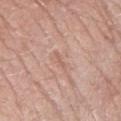Q: Was a biopsy performed?
A: no biopsy performed (imaged during a skin exam)
Q: What lighting was used for the tile?
A: white-light
Q: Where on the body is the lesion?
A: the right forearm
Q: How large is the lesion?
A: ≈2.5 mm
Q: What kind of image is this?
A: total-body-photography crop, ~15 mm field of view
Q: What did automated image analysis measure?
A: an area of roughly 2 mm², an eccentricity of roughly 0.95, and a symmetry-axis asymmetry near 0.45; internal color variation of about 0 on a 0–10 scale and a peripheral color-asymmetry measure near 0
Q: Who is the patient?
A: female, about 55 years old